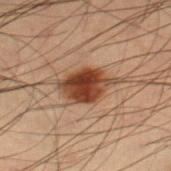Imaged during a routine full-body skin examination; the lesion was not biopsied and no histopathology is available. The total-body-photography lesion software estimated an eccentricity of roughly 0.75 and a symmetry-axis asymmetry near 0.2. Captured under cross-polarized illumination. This image is a 15 mm lesion crop taken from a total-body photograph. A male subject, approximately 55 years of age. The lesion is on the right thigh.